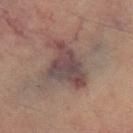Captured during whole-body skin photography for melanoma surveillance; the lesion was not biopsied. Cropped from a whole-body photographic skin survey; the tile spans about 15 mm. Captured under cross-polarized illumination. The lesion's longest dimension is about 6 mm. The patient is a female aged 68–72. The lesion is located on the right thigh.